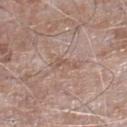Clinical impression: The lesion was photographed on a routine skin check and not biopsied; there is no pathology result. Context: The total-body-photography lesion software estimated a lesion area of about 3 mm², a shape eccentricity near 0.9, and two-axis asymmetry of about 0.45. The analysis additionally found border irregularity of about 5.5 on a 0–10 scale and a peripheral color-asymmetry measure near 0. Cropped from a whole-body photographic skin survey; the tile spans about 15 mm. The lesion is on the right lower leg. A male subject, roughly 75 years of age. Captured under white-light illumination. Longest diameter approximately 3 mm.